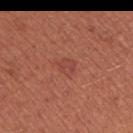The lesion was tiled from a total-body skin photograph and was not biopsied. The patient is a female approximately 35 years of age. Approximately 2.5 mm at its widest. A 15 mm crop from a total-body photograph taken for skin-cancer surveillance. Captured under white-light illumination. Automated tile analysis of the lesion measured a lesion area of about 4 mm², an eccentricity of roughly 0.6, and a symmetry-axis asymmetry near 0.25. And it measured a mean CIELAB color near L≈46 a*≈28 b*≈29 and a lesion-to-skin contrast of about 4.5 (normalized; higher = more distinct). Located on the left upper arm.